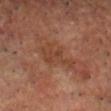Q: Was a biopsy performed?
A: total-body-photography surveillance lesion; no biopsy